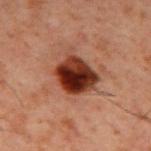No biopsy was performed on this lesion — it was imaged during a full skin examination and was not determined to be concerning. This is a cross-polarized tile. A region of skin cropped from a whole-body photographic capture, roughly 15 mm wide. Approximately 4.5 mm at its widest. A male subject, aged 58–62. The lesion is located on the mid back.